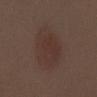Q: What kind of image is this?
A: 15 mm crop, total-body photography
Q: What did automated image analysis measure?
A: a lesion color around L≈33 a*≈15 b*≈20 in CIELAB, roughly 5 lightness units darker than nearby skin, and a lesion-to-skin contrast of about 5 (normalized; higher = more distinct); a border-irregularity rating of about 2/10, a within-lesion color-variation index near 2/10, and peripheral color asymmetry of about 0.5; an automated nevus-likeness rating near 90 out of 100 and lesion-presence confidence of about 100/100
Q: Where on the body is the lesion?
A: the mid back
Q: How was the tile lit?
A: white-light illumination
Q: How large is the lesion?
A: ≈6.5 mm
Q: What are the patient's age and sex?
A: female, approximately 50 years of age